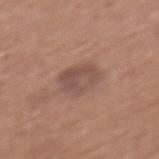notes = no biopsy performed (imaged during a skin exam).